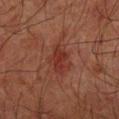Q: What is the imaging modality?
A: 15 mm crop, total-body photography
Q: What are the patient's age and sex?
A: male, aged 63–67
Q: What is the anatomic site?
A: the left forearm
Q: How large is the lesion?
A: about 2.5 mm